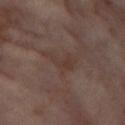Impression: This lesion was catalogued during total-body skin photography and was not selected for biopsy. Background: A 15 mm close-up extracted from a 3D total-body photography capture. The tile uses cross-polarized illumination. The subject is a female in their mid-50s. The lesion is located on the leg. The recorded lesion diameter is about 3.5 mm.